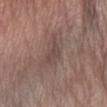biopsy status: no biopsy performed (imaged during a skin exam) | image source: 15 mm crop, total-body photography | anatomic site: the left forearm | patient: female, in their mid-60s | tile lighting: white-light | automated metrics: a lesion color around L≈45 a*≈15 b*≈21 in CIELAB, roughly 7 lightness units darker than nearby skin, and a normalized border contrast of about 5.5.